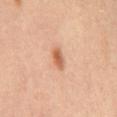Case summary:
– follow-up — no biopsy performed (imaged during a skin exam)
– lesion diameter — about 2.5 mm
– image source — total-body-photography crop, ~15 mm field of view
– anatomic site — the mid back
– illumination — cross-polarized
– automated metrics — a shape eccentricity near 0.85 and a symmetry-axis asymmetry near 0.35; a nevus-likeness score of about 90/100
– subject — male, aged 58 to 62